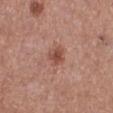Clinical impression:
This lesion was catalogued during total-body skin photography and was not selected for biopsy.
Image and clinical context:
The total-body-photography lesion software estimated a footprint of about 5 mm², a shape eccentricity near 0.35, and a symmetry-axis asymmetry near 0.3. And it measured an average lesion color of about L≈50 a*≈24 b*≈28 (CIELAB), roughly 9 lightness units darker than nearby skin, and a lesion-to-skin contrast of about 7 (normalized; higher = more distinct). The analysis additionally found radial color variation of about 1. The analysis additionally found a detector confidence of about 100 out of 100 that the crop contains a lesion. A female subject approximately 60 years of age. About 2.5 mm across. Located on the right lower leg. This is a white-light tile. Cropped from a whole-body photographic skin survey; the tile spans about 15 mm.